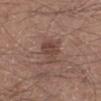Part of a total-body skin-imaging series; this lesion was reviewed on a skin check and was not flagged for biopsy.
This is a white-light tile.
The lesion is located on the left lower leg.
The total-body-photography lesion software estimated an area of roughly 7 mm², an eccentricity of roughly 0.65, and a symmetry-axis asymmetry near 0.35. The software also gave an automated nevus-likeness rating near 25 out of 100 and a detector confidence of about 100 out of 100 that the crop contains a lesion.
Approximately 3.5 mm at its widest.
A roughly 15 mm field-of-view crop from a total-body skin photograph.
A male patient, aged 18–22.